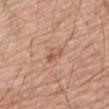workup = no biopsy performed (imaged during a skin exam)
body site = the back
subject = male, aged approximately 80
lesion size = about 3.5 mm
illumination = white-light
automated lesion analysis = a lesion area of about 3.5 mm², an eccentricity of roughly 0.9, and two-axis asymmetry of about 0.35
image source = ~15 mm crop, total-body skin-cancer survey